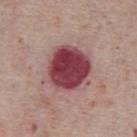Imaged during a routine full-body skin examination; the lesion was not biopsied and no histopathology is available.
This image is a 15 mm lesion crop taken from a total-body photograph.
The lesion is located on the chest.
This is a white-light tile.
Approximately 5 mm at its widest.
A male patient in their mid-70s.